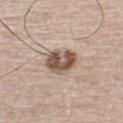biopsy_status: not biopsied; imaged during a skin examination
patient:
  sex: male
  age_approx: 65
lesion_size:
  long_diameter_mm_approx: 3.5
lighting: white-light
image:
  source: total-body photography crop
  field_of_view_mm: 15
site: left thigh
automated_metrics:
  area_mm2_approx: 9.5
  shape_asymmetry: 0.15
  border_irregularity_0_10: 1.5
  color_variation_0_10: 6.5